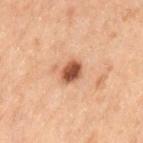| key | value |
|---|---|
| workup | total-body-photography surveillance lesion; no biopsy |
| location | the lower back |
| imaging modality | 15 mm crop, total-body photography |
| diameter | about 2.5 mm |
| lighting | cross-polarized illumination |
| subject | male, aged around 70 |